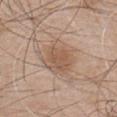Part of a total-body skin-imaging series; this lesion was reviewed on a skin check and was not flagged for biopsy.
A 15 mm close-up extracted from a 3D total-body photography capture.
A male subject aged 43–47.
On the front of the torso.
The recorded lesion diameter is about 4.5 mm.